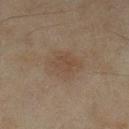Notes:
- automated lesion analysis · an area of roughly 14 mm²; a mean CIELAB color near L≈42 a*≈13 b*≈25, a lesion–skin lightness drop of about 5, and a normalized border contrast of about 5; border irregularity of about 2 on a 0–10 scale, internal color variation of about 2.5 on a 0–10 scale, and radial color variation of about 0.5
- image source · 15 mm crop, total-body photography
- tile lighting · cross-polarized
- patient · female, aged around 60
- body site · the left lower leg